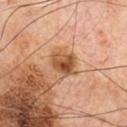{"biopsy_status": "not biopsied; imaged during a skin examination", "automated_metrics": {"area_mm2_approx": 8.0, "shape_asymmetry": 0.2, "cielab_L": 51, "cielab_a": 22, "cielab_b": 36, "vs_skin_contrast_norm": 9.5}, "site": "chest", "image": {"source": "total-body photography crop", "field_of_view_mm": 15}, "patient": {"sex": "male", "age_approx": 70}}